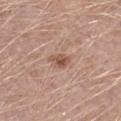Part of a total-body skin-imaging series; this lesion was reviewed on a skin check and was not flagged for biopsy.